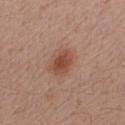Case summary:
• biopsy status: total-body-photography surveillance lesion; no biopsy
• lighting: white-light
• image-analysis metrics: border irregularity of about 1.5 on a 0–10 scale, internal color variation of about 2.5 on a 0–10 scale, and peripheral color asymmetry of about 0.5
• site: the right forearm
• subject: male, aged around 30
• imaging modality: 15 mm crop, total-body photography